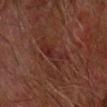Part of a total-body skin-imaging series; this lesion was reviewed on a skin check and was not flagged for biopsy. From the left forearm. The tile uses cross-polarized illumination. An algorithmic analysis of the crop reported an area of roughly 7.5 mm², an eccentricity of roughly 0.9, and a symmetry-axis asymmetry near 0.45. The software also gave a mean CIELAB color near L≈22 a*≈20 b*≈19, about 4 CIELAB-L* units darker than the surrounding skin, and a normalized lesion–skin contrast near 5.5. The software also gave a border-irregularity rating of about 5.5/10. And it measured lesion-presence confidence of about 95/100. A male patient, aged approximately 75. The recorded lesion diameter is about 5 mm. A lesion tile, about 15 mm wide, cut from a 3D total-body photograph.